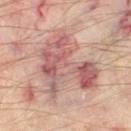biopsy status = no biopsy performed (imaged during a skin exam), image-analysis metrics = a mean CIELAB color near L≈56 a*≈24 b*≈22 and roughly 11 lightness units darker than nearby skin, acquisition = ~15 mm tile from a whole-body skin photo, subject = aged 53–57, lighting = cross-polarized illumination, site = the left thigh.